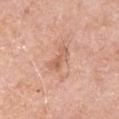A close-up tile cropped from a whole-body skin photograph, about 15 mm across. Longest diameter approximately 3.5 mm. A male patient, aged around 60. The lesion is on the right upper arm.